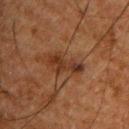workup = imaged on a skin check; not biopsied | acquisition = ~15 mm tile from a whole-body skin photo | body site = the upper back | patient = male, aged 48–52.